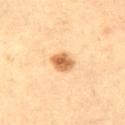patient: male, aged 58–62 | image: ~15 mm tile from a whole-body skin photo.